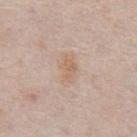{
  "biopsy_status": "not biopsied; imaged during a skin examination",
  "lighting": "white-light",
  "patient": {
    "sex": "male",
    "age_approx": 75
  },
  "automated_metrics": {
    "cielab_L": 63,
    "cielab_a": 16,
    "cielab_b": 29,
    "vs_skin_darker_L": 6.0,
    "vs_skin_contrast_norm": 5.5,
    "border_irregularity_0_10": 2.5,
    "color_variation_0_10": 2.0,
    "nevus_likeness_0_100": 10
  },
  "lesion_size": {
    "long_diameter_mm_approx": 3.5
  },
  "site": "chest",
  "image": {
    "source": "total-body photography crop",
    "field_of_view_mm": 15
  }
}